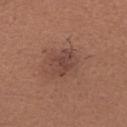Impression:
Captured during whole-body skin photography for melanoma surveillance; the lesion was not biopsied.
Background:
Imaged with white-light lighting. Located on the left forearm. A roughly 15 mm field-of-view crop from a total-body skin photograph. About 3.5 mm across. The subject is a female about 40 years old.